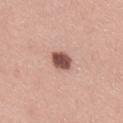biopsy status: catalogued during a skin exam; not biopsied | subject: female, about 35 years old | acquisition: 15 mm crop, total-body photography | body site: the right thigh | automated lesion analysis: an area of roughly 5.5 mm², an eccentricity of roughly 0.7, and a shape-asymmetry score of about 0.2 (0 = symmetric); a border-irregularity rating of about 2/10, internal color variation of about 3.5 on a 0–10 scale, and a peripheral color-asymmetry measure near 1 | lighting: white-light illumination | lesion size: about 3 mm.